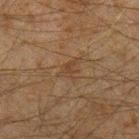The lesion was photographed on a routine skin check and not biopsied; there is no pathology result.
A male subject, in their mid- to late 30s.
A region of skin cropped from a whole-body photographic capture, roughly 15 mm wide.
On the left forearm.